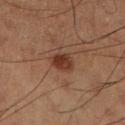workup: no biopsy performed (imaged during a skin exam)
body site: the right lower leg
acquisition: total-body-photography crop, ~15 mm field of view
patient: male, aged 58 to 62
lighting: cross-polarized
lesion diameter: about 3 mm